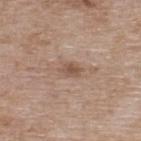<lesion>
<biopsy_status>not biopsied; imaged during a skin examination</biopsy_status>
<automated_metrics>
  <cielab_L>53</cielab_L>
  <cielab_a>18</cielab_a>
  <cielab_b>27</cielab_b>
  <vs_skin_darker_L>9.0</vs_skin_darker_L>
  <vs_skin_contrast_norm>6.5</vs_skin_contrast_norm>
  <border_irregularity_0_10>2.5</border_irregularity_0_10>
  <color_variation_0_10>2.5</color_variation_0_10>
</automated_metrics>
<site>upper back</site>
<patient>
  <sex>female</sex>
  <age_approx>75</age_approx>
</patient>
<lesion_size>
  <long_diameter_mm_approx>2.5</long_diameter_mm_approx>
</lesion_size>
<lighting>white-light</lighting>
<image>
  <source>total-body photography crop</source>
  <field_of_view_mm>15</field_of_view_mm>
</image>
</lesion>